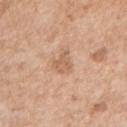Notes:
– biopsy status · total-body-photography surveillance lesion; no biopsy
– image-analysis metrics · a footprint of about 5.5 mm², a shape eccentricity near 0.8, and a shape-asymmetry score of about 0.4 (0 = symmetric); a lesion–skin lightness drop of about 8 and a normalized lesion–skin contrast near 5.5
– lighting · white-light illumination
– patient · male, about 60 years old
– image source · ~15 mm tile from a whole-body skin photo
– body site · the right upper arm